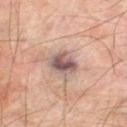Imaged during a routine full-body skin examination; the lesion was not biopsied and no histopathology is available. A region of skin cropped from a whole-body photographic capture, roughly 15 mm wide. The lesion is located on the leg. The subject is a male aged approximately 70.